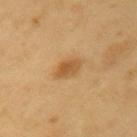workup: catalogued during a skin exam; not biopsied | TBP lesion metrics: a lesion area of about 4.5 mm² and an eccentricity of roughly 0.75; a lesion color around L≈54 a*≈20 b*≈41 in CIELAB, roughly 10 lightness units darker than nearby skin, and a lesion-to-skin contrast of about 7.5 (normalized; higher = more distinct); a within-lesion color-variation index near 2.5/10 and radial color variation of about 1; a classifier nevus-likeness of about 85/100 and a detector confidence of about 100 out of 100 that the crop contains a lesion | location: the left upper arm | image source: ~15 mm crop, total-body skin-cancer survey | subject: male, approximately 60 years of age | diameter: ≈3 mm | illumination: cross-polarized.